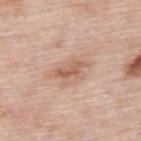biopsy_status: not biopsied; imaged during a skin examination
patient:
  sex: male
  age_approx: 55
image:
  source: total-body photography crop
  field_of_view_mm: 15
lighting: white-light
lesion_size:
  long_diameter_mm_approx: 3.0
site: upper back